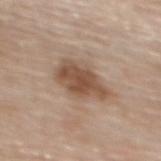Q: Lesion size?
A: about 6 mm
Q: What are the patient's age and sex?
A: female, approximately 65 years of age
Q: What is the anatomic site?
A: the upper back
Q: How was this image acquired?
A: 15 mm crop, total-body photography